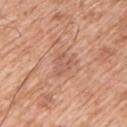This lesion was catalogued during total-body skin photography and was not selected for biopsy. The lesion is on the left upper arm. Automated image analysis of the tile measured an outline eccentricity of about 0.6 (0 = round, 1 = elongated). A male subject, aged approximately 60. About 3 mm across. Captured under white-light illumination. A close-up tile cropped from a whole-body skin photograph, about 15 mm across.